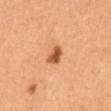Q: Is there a histopathology result?
A: total-body-photography surveillance lesion; no biopsy
Q: What kind of image is this?
A: ~15 mm tile from a whole-body skin photo
Q: Illumination type?
A: cross-polarized illumination
Q: Patient demographics?
A: female
Q: Automated lesion metrics?
A: border irregularity of about 2.5 on a 0–10 scale and peripheral color asymmetry of about 1; a nevus-likeness score of about 95/100 and lesion-presence confidence of about 100/100
Q: Where on the body is the lesion?
A: the abdomen
Q: Lesion size?
A: about 2.5 mm